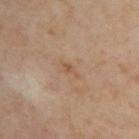Case summary:
- notes: imaged on a skin check; not biopsied
- body site: the upper back
- imaging modality: ~15 mm crop, total-body skin-cancer survey
- patient: in their mid- to late 50s